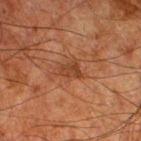follow-up = imaged on a skin check; not biopsied | site = the right thigh | diameter = ≈2.5 mm | patient = male, aged approximately 80 | imaging modality = total-body-photography crop, ~15 mm field of view | illumination = cross-polarized.